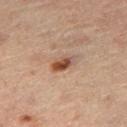<case>
<site>right thigh</site>
<patient>
  <sex>male</sex>
  <age_approx>55</age_approx>
</patient>
<lighting>cross-polarized</lighting>
<lesion_size>
  <long_diameter_mm_approx>3.5</long_diameter_mm_approx>
</lesion_size>
<image>
  <source>total-body photography crop</source>
  <field_of_view_mm>15</field_of_view_mm>
</image>
</case>